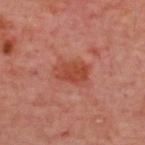Image and clinical context:
The total-body-photography lesion software estimated a footprint of about 9 mm², an outline eccentricity of about 0.75 (0 = round, 1 = elongated), and two-axis asymmetry of about 0.2. The recorded lesion diameter is about 4 mm. Imaged with cross-polarized lighting. The subject is aged approximately 55. This image is a 15 mm lesion crop taken from a total-body photograph. On the upper back.